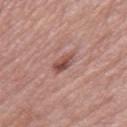Captured during whole-body skin photography for melanoma surveillance; the lesion was not biopsied. A region of skin cropped from a whole-body photographic capture, roughly 15 mm wide. A female subject in their mid- to late 50s. On the right thigh.